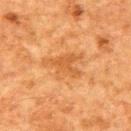Findings:
- follow-up · catalogued during a skin exam; not biopsied
- lighting · cross-polarized
- subject · male, aged 78–82
- acquisition · ~15 mm crop, total-body skin-cancer survey
- lesion size · ~5.5 mm (longest diameter)
- anatomic site · the upper back